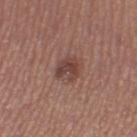Imaged during a routine full-body skin examination; the lesion was not biopsied and no histopathology is available.